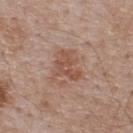follow-up — catalogued during a skin exam; not biopsied | patient — male, aged around 55 | lighting — white-light illumination | imaging modality — total-body-photography crop, ~15 mm field of view | lesion size — ~4 mm (longest diameter) | site — the upper back.